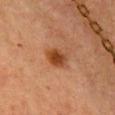This lesion was catalogued during total-body skin photography and was not selected for biopsy. The recorded lesion diameter is about 3 mm. A lesion tile, about 15 mm wide, cut from a 3D total-body photograph. Imaged with cross-polarized lighting. The lesion-visualizer software estimated a lesion color around L≈37 a*≈22 b*≈33 in CIELAB, a lesion–skin lightness drop of about 10, and a lesion-to-skin contrast of about 9.5 (normalized; higher = more distinct). The software also gave a border-irregularity index near 2/10, internal color variation of about 4.5 on a 0–10 scale, and a peripheral color-asymmetry measure near 1.5. Located on the chest. A female subject, approximately 40 years of age.